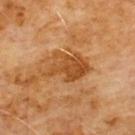biopsy_status: not biopsied; imaged during a skin examination
lesion_size:
  long_diameter_mm_approx: 5.5
patient:
  sex: male
  age_approx: 60
site: chest
image:
  source: total-body photography crop
  field_of_view_mm: 15
lighting: cross-polarized